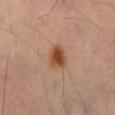Case summary:
- workup: catalogued during a skin exam; not biopsied